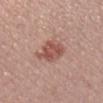{
  "biopsy_status": "not biopsied; imaged during a skin examination",
  "lesion_size": {
    "long_diameter_mm_approx": 4.0
  },
  "image": {
    "source": "total-body photography crop",
    "field_of_view_mm": 15
  },
  "lighting": "white-light",
  "automated_metrics": {
    "eccentricity": 0.6,
    "shape_asymmetry": 0.2
  },
  "patient": {
    "sex": "male",
    "age_approx": 30
  }
}